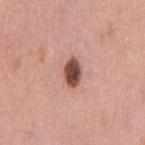Clinical summary: The lesion is located on the leg. The tile uses white-light illumination. A female patient aged approximately 50. Measured at roughly 3.5 mm in maximum diameter. The total-body-photography lesion software estimated an average lesion color of about L≈49 a*≈24 b*≈24 (CIELAB), roughly 19 lightness units darker than nearby skin, and a lesion-to-skin contrast of about 12.5 (normalized; higher = more distinct). And it measured a border-irregularity index near 2/10 and a within-lesion color-variation index near 4.5/10. The software also gave a classifier nevus-likeness of about 100/100 and a lesion-detection confidence of about 100/100. A 15 mm close-up extracted from a 3D total-body photography capture.